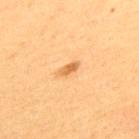workup: catalogued during a skin exam; not biopsied
imaging modality: total-body-photography crop, ~15 mm field of view
diameter: ~2.5 mm (longest diameter)
site: the upper back
automated lesion analysis: an average lesion color of about L≈59 a*≈22 b*≈44 (CIELAB), a lesion–skin lightness drop of about 11, and a lesion-to-skin contrast of about 7.5 (normalized; higher = more distinct); a border-irregularity rating of about 2.5/10, a within-lesion color-variation index near 0.5/10, and peripheral color asymmetry of about 0; an automated nevus-likeness rating near 80 out of 100
patient: male, aged around 55
tile lighting: cross-polarized